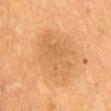  biopsy_status: not biopsied; imaged during a skin examination
  site: back
  image:
    source: total-body photography crop
    field_of_view_mm: 15
  patient:
    sex: male
    age_approx: 50